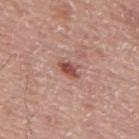The patient is a male aged 73–77.
A region of skin cropped from a whole-body photographic capture, roughly 15 mm wide.
Located on the mid back.